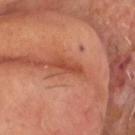Clinical impression: Captured during whole-body skin photography for melanoma surveillance; the lesion was not biopsied. Acquisition and patient details: A male subject aged 58 to 62. Automated tile analysis of the lesion measured a mean CIELAB color near L≈45 a*≈30 b*≈34 and a normalized border contrast of about 7.5. And it measured peripheral color asymmetry of about 0. A 15 mm crop from a total-body photograph taken for skin-cancer surveillance. The lesion is located on the head or neck.